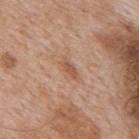Assessment: This lesion was catalogued during total-body skin photography and was not selected for biopsy.